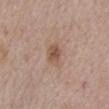Imaged during a routine full-body skin examination; the lesion was not biopsied and no histopathology is available. The tile uses white-light illumination. A close-up tile cropped from a whole-body skin photograph, about 15 mm across. The patient is a male about 60 years old. The lesion's longest dimension is about 3.5 mm. The lesion is located on the abdomen.